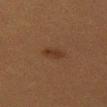Context: The recorded lesion diameter is about 2.5 mm. A 15 mm close-up tile from a total-body photography series done for melanoma screening. A female subject aged approximately 40. The tile uses cross-polarized illumination. On the leg.